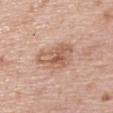Notes:
– notes: no biopsy performed (imaged during a skin exam)
– image: total-body-photography crop, ~15 mm field of view
– subject: female, roughly 50 years of age
– illumination: white-light illumination
– lesion size: about 5 mm
– body site: the upper back